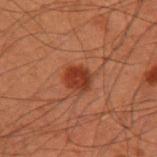Part of a total-body skin-imaging series; this lesion was reviewed on a skin check and was not flagged for biopsy. A roughly 15 mm field-of-view crop from a total-body skin photograph. Located on the left upper arm. The subject is a male in their 60s. This is a cross-polarized tile. Automated tile analysis of the lesion measured a border-irregularity index near 2/10 and a peripheral color-asymmetry measure near 1.